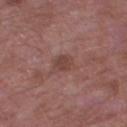- follow-up: total-body-photography surveillance lesion; no biopsy
- image source: 15 mm crop, total-body photography
- subject: male, in their mid- to late 50s
- diameter: ≈3 mm
- site: the leg
- illumination: white-light illumination